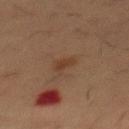Clinical impression: Recorded during total-body skin imaging; not selected for excision or biopsy. Image and clinical context: Captured under cross-polarized illumination. A lesion tile, about 15 mm wide, cut from a 3D total-body photograph. A male patient about 55 years old. From the chest. Approximately 2.5 mm at its widest.